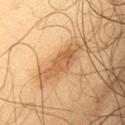Part of a total-body skin-imaging series; this lesion was reviewed on a skin check and was not flagged for biopsy.
A close-up tile cropped from a whole-body skin photograph, about 15 mm across.
A male subject aged approximately 65.
The lesion is on the left upper arm.